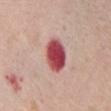The lesion was photographed on a routine skin check and not biopsied; there is no pathology result.
A female patient in their mid- to late 70s.
From the chest.
A lesion tile, about 15 mm wide, cut from a 3D total-body photograph.
The tile uses white-light illumination.
The lesion's longest dimension is about 4 mm.
The total-body-photography lesion software estimated a mean CIELAB color near L≈49 a*≈36 b*≈22 and a normalized border contrast of about 13.5. And it measured a border-irregularity index near 1/10 and a within-lesion color-variation index near 5/10.